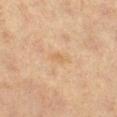<record>
  <biopsy_status>not biopsied; imaged during a skin examination</biopsy_status>
  <patient>
    <sex>female</sex>
    <age_approx>40</age_approx>
  </patient>
  <image>
    <source>total-body photography crop</source>
    <field_of_view_mm>15</field_of_view_mm>
  </image>
  <site>left lower leg</site>
</record>